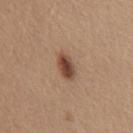This image is a 15 mm lesion crop taken from a total-body photograph. Longest diameter approximately 3.5 mm. Located on the abdomen. A female patient, in their mid-40s.